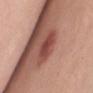The lesion was tiled from a total-body skin photograph and was not biopsied. The lesion is on the chest. This is a white-light tile. The lesion-visualizer software estimated a lesion area of about 6.5 mm² and a symmetry-axis asymmetry near 0.2. And it measured a nevus-likeness score of about 30/100. A 15 mm close-up tile from a total-body photography series done for melanoma screening. A female subject aged around 45.